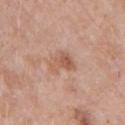| feature | finding |
|---|---|
| follow-up | total-body-photography surveillance lesion; no biopsy |
| location | the right upper arm |
| subject | female, aged approximately 70 |
| image source | ~15 mm crop, total-body skin-cancer survey |